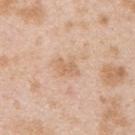Clinical impression: Captured during whole-body skin photography for melanoma surveillance; the lesion was not biopsied. Acquisition and patient details: This is a white-light tile. A male patient approximately 25 years of age. Longest diameter approximately 2.5 mm. A roughly 15 mm field-of-view crop from a total-body skin photograph. Automated image analysis of the tile measured a mean CIELAB color near L≈65 a*≈19 b*≈34, a lesion–skin lightness drop of about 8, and a normalized border contrast of about 5.5. From the upper back.